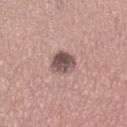<record>
<biopsy_status>not biopsied; imaged during a skin examination</biopsy_status>
<image>
  <source>total-body photography crop</source>
  <field_of_view_mm>15</field_of_view_mm>
</image>
<lighting>white-light</lighting>
<automated_metrics>
  <area_mm2_approx>7.0</area_mm2_approx>
  <eccentricity>0.5</eccentricity>
  <shape_asymmetry>0.2</shape_asymmetry>
  <border_irregularity_0_10>2.0</border_irregularity_0_10>
  <color_variation_0_10>7.0</color_variation_0_10>
  <nevus_likeness_0_100>10</nevus_likeness_0_100>
  <lesion_detection_confidence_0_100>100</lesion_detection_confidence_0_100>
</automated_metrics>
<lesion_size>
  <long_diameter_mm_approx>3.0</long_diameter_mm_approx>
</lesion_size>
<patient>
  <sex>female</sex>
  <age_approx>60</age_approx>
</patient>
<site>right thigh</site>
</record>